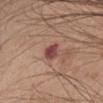Assessment: Recorded during total-body skin imaging; not selected for excision or biopsy. Image and clinical context: This image is a 15 mm lesion crop taken from a total-body photograph. A male subject, roughly 65 years of age. Automated image analysis of the tile measured a footprint of about 4 mm², an eccentricity of roughly 0.75, and two-axis asymmetry of about 0.25. The lesion is on the chest. Approximately 2.5 mm at its widest. Captured under white-light illumination.